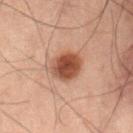Impression: No biopsy was performed on this lesion — it was imaged during a full skin examination and was not determined to be concerning. Acquisition and patient details: A male patient about 60 years old. On the abdomen. This image is a 15 mm lesion crop taken from a total-body photograph.